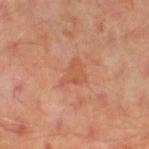<tbp_lesion>
<lesion_size>
  <long_diameter_mm_approx>3.5</long_diameter_mm_approx>
</lesion_size>
<site>left leg</site>
<patient>
  <sex>male</sex>
  <age_approx>50</age_approx>
</patient>
<image>
  <source>total-body photography crop</source>
  <field_of_view_mm>15</field_of_view_mm>
</image>
</tbp_lesion>